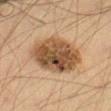Imaged during a routine full-body skin examination; the lesion was not biopsied and no histopathology is available.
Automated image analysis of the tile measured an area of roughly 26 mm², an outline eccentricity of about 0.65 (0 = round, 1 = elongated), and a symmetry-axis asymmetry near 0.15. It also reported a mean CIELAB color near L≈42 a*≈15 b*≈29, about 13 CIELAB-L* units darker than the surrounding skin, and a lesion-to-skin contrast of about 10.5 (normalized; higher = more distinct). It also reported a border-irregularity rating of about 1.5/10 and internal color variation of about 7 on a 0–10 scale. And it measured a nevus-likeness score of about 40/100 and lesion-presence confidence of about 100/100.
A close-up tile cropped from a whole-body skin photograph, about 15 mm across.
The tile uses cross-polarized illumination.
The lesion is located on the right thigh.
Longest diameter approximately 6.5 mm.
A male patient, approximately 60 years of age.